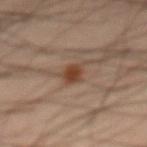<tbp_lesion>
  <biopsy_status>not biopsied; imaged during a skin examination</biopsy_status>
  <image>
    <source>total-body photography crop</source>
    <field_of_view_mm>15</field_of_view_mm>
  </image>
  <patient>
    <sex>male</sex>
    <age_approx>60</age_approx>
  </patient>
  <lighting>cross-polarized</lighting>
  <site>abdomen</site>
</tbp_lesion>